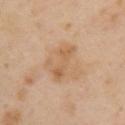Impression:
Imaged during a routine full-body skin examination; the lesion was not biopsied and no histopathology is available.
Acquisition and patient details:
On the left upper arm. The recorded lesion diameter is about 4.5 mm. Cropped from a whole-body photographic skin survey; the tile spans about 15 mm. A female patient in their 40s.